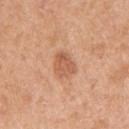| feature | finding |
|---|---|
| notes | catalogued during a skin exam; not biopsied |
| body site | the right upper arm |
| imaging modality | 15 mm crop, total-body photography |
| patient | female, aged 38–42 |
| size | ~3.5 mm (longest diameter) |
| lighting | white-light illumination |